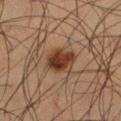This is a cross-polarized tile.
The lesion is located on the leg.
Measured at roughly 3.5 mm in maximum diameter.
The total-body-photography lesion software estimated an average lesion color of about L≈29 a*≈17 b*≈25 (CIELAB), roughly 11 lightness units darker than nearby skin, and a lesion-to-skin contrast of about 11 (normalized; higher = more distinct). The analysis additionally found a border-irregularity index near 2/10, a within-lesion color-variation index near 4.5/10, and a peripheral color-asymmetry measure near 1.5.
A roughly 15 mm field-of-view crop from a total-body skin photograph.
A male patient, in their 50s.